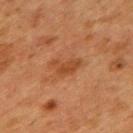workup = total-body-photography surveillance lesion; no biopsy | body site = the mid back | subject = female, about 40 years old | image = ~15 mm crop, total-body skin-cancer survey.